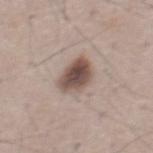About 4 mm across. The subject is a male in their mid-50s. The lesion-visualizer software estimated a mean CIELAB color near L≈50 a*≈15 b*≈22, a lesion–skin lightness drop of about 15, and a normalized border contrast of about 10.5. And it measured a lesion-detection confidence of about 100/100. Imaged with white-light lighting. A 15 mm close-up tile from a total-body photography series done for melanoma screening.